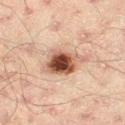{
  "biopsy_status": "not biopsied; imaged during a skin examination",
  "patient": {
    "sex": "male",
    "age_approx": 45
  },
  "image": {
    "source": "total-body photography crop",
    "field_of_view_mm": 15
  },
  "site": "right thigh"
}